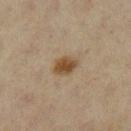The lesion was tiled from a total-body skin photograph and was not biopsied. The recorded lesion diameter is about 3 mm. The tile uses cross-polarized illumination. The patient is a female aged around 65. A roughly 15 mm field-of-view crop from a total-body skin photograph. Located on the leg.